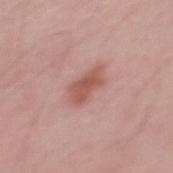{
  "biopsy_status": "not biopsied; imaged during a skin examination",
  "image": {
    "source": "total-body photography crop",
    "field_of_view_mm": 15
  },
  "patient": {
    "sex": "male",
    "age_approx": 30
  },
  "site": "back",
  "lighting": "white-light",
  "automated_metrics": {
    "area_mm2_approx": 7.5,
    "eccentricity": 0.85,
    "shape_asymmetry": 0.25,
    "border_irregularity_0_10": 3.0,
    "color_variation_0_10": 2.5,
    "nevus_likeness_0_100": 55,
    "lesion_detection_confidence_0_100": 100
  }
}